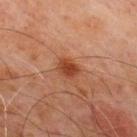Assessment:
The lesion was tiled from a total-body skin photograph and was not biopsied.
Context:
Measured at roughly 2.5 mm in maximum diameter. A male patient aged approximately 70. Located on the chest. Cropped from a whole-body photographic skin survey; the tile spans about 15 mm. The lesion-visualizer software estimated an outline eccentricity of about 0.7 (0 = round, 1 = elongated) and a symmetry-axis asymmetry near 0.2. The analysis additionally found a lesion color around L≈36 a*≈24 b*≈30 in CIELAB. The analysis additionally found a border-irregularity rating of about 2/10 and peripheral color asymmetry of about 1. This is a cross-polarized tile.